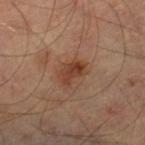<record>
<biopsy_status>not biopsied; imaged during a skin examination</biopsy_status>
<image>
  <source>total-body photography crop</source>
  <field_of_view_mm>15</field_of_view_mm>
</image>
<automated_metrics>
  <cielab_L>41</cielab_L>
  <cielab_a>23</cielab_a>
  <cielab_b>31</cielab_b>
  <vs_skin_darker_L>10.0</vs_skin_darker_L>
  <border_irregularity_0_10>3.0</border_irregularity_0_10>
  <color_variation_0_10>4.5</color_variation_0_10>
  <peripheral_color_asymmetry>2.0</peripheral_color_asymmetry>
  <lesion_detection_confidence_0_100>100</lesion_detection_confidence_0_100>
</automated_metrics>
<site>right thigh</site>
<lesion_size>
  <long_diameter_mm_approx>3.0</long_diameter_mm_approx>
</lesion_size>
</record>